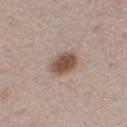{"biopsy_status": "not biopsied; imaged during a skin examination", "image": {"source": "total-body photography crop", "field_of_view_mm": 15}, "lesion_size": {"long_diameter_mm_approx": 3.5}, "patient": {"sex": "male", "age_approx": 65}, "automated_metrics": {"area_mm2_approx": 7.5, "eccentricity": 0.65, "shape_asymmetry": 0.2, "cielab_L": 51, "cielab_a": 17, "cielab_b": 26, "vs_skin_darker_L": 14.0, "vs_skin_contrast_norm": 10.0, "border_irregularity_0_10": 1.5, "color_variation_0_10": 3.5, "peripheral_color_asymmetry": 1.0}, "lighting": "white-light", "site": "right thigh"}